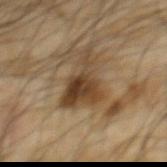workup — total-body-photography surveillance lesion; no biopsy | image source — 15 mm crop, total-body photography | subject — male, aged approximately 65 | anatomic site — the upper back.